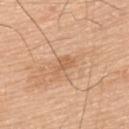A male patient aged 78 to 82.
A close-up tile cropped from a whole-body skin photograph, about 15 mm across.
On the upper back.
The lesion's longest dimension is about 2.5 mm.
Imaged with white-light lighting.